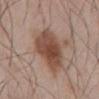biopsy_status: not biopsied; imaged during a skin examination
lesion_size:
  long_diameter_mm_approx: 5.5
image:
  source: total-body photography crop
  field_of_view_mm: 15
site: abdomen
lighting: white-light
patient:
  sex: male
  age_approx: 55